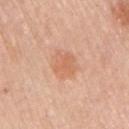follow-up: total-body-photography surveillance lesion; no biopsy
diameter: ~3 mm (longest diameter)
location: the right upper arm
subject: female, aged approximately 65
illumination: white-light
image source: total-body-photography crop, ~15 mm field of view